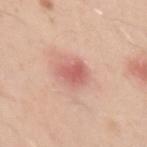The lesion was photographed on a routine skin check and not biopsied; there is no pathology result. A male subject aged around 30. Automated tile analysis of the lesion measured a border-irregularity rating of about 2.5/10, internal color variation of about 3 on a 0–10 scale, and a peripheral color-asymmetry measure near 1. It also reported a classifier nevus-likeness of about 0/100. This is a white-light tile. A 15 mm close-up extracted from a 3D total-body photography capture. From the left upper arm. The lesion's longest dimension is about 3.5 mm.